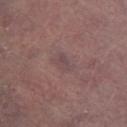Notes:
• notes · imaged on a skin check; not biopsied
• location · the left lower leg
• image source · 15 mm crop, total-body photography
• subject · male, approximately 65 years of age